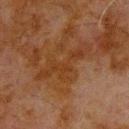The lesion's longest dimension is about 7.5 mm. Cropped from a whole-body photographic skin survey; the tile spans about 15 mm. From the upper back. A male patient, approximately 80 years of age.